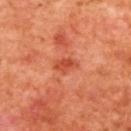{
  "biopsy_status": "not biopsied; imaged during a skin examination",
  "lesion_size": {
    "long_diameter_mm_approx": 2.5
  },
  "image": {
    "source": "total-body photography crop",
    "field_of_view_mm": 15
  },
  "lighting": "cross-polarized",
  "site": "upper back",
  "patient": {
    "sex": "female",
    "age_approx": 40
  }
}